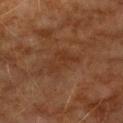Approximately 4 mm at its widest. Imaged with cross-polarized lighting. A male subject aged around 60. The lesion is located on the left upper arm. Cropped from a whole-body photographic skin survey; the tile spans about 15 mm.